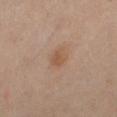Cropped from a whole-body photographic skin survey; the tile spans about 15 mm.
A female subject, about 50 years old.
From the leg.